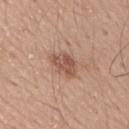Case summary:
- biopsy status · no biopsy performed (imaged during a skin exam)
- acquisition · ~15 mm tile from a whole-body skin photo
- patient · male, roughly 20 years of age
- body site · the right upper arm